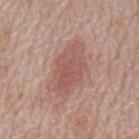Impression:
The lesion was tiled from a total-body skin photograph and was not biopsied.
Clinical summary:
The lesion's longest dimension is about 6.5 mm. On the mid back. Imaged with white-light lighting. A 15 mm crop from a total-body photograph taken for skin-cancer surveillance. The lesion-visualizer software estimated a shape eccentricity near 0.85 and a symmetry-axis asymmetry near 0.2. The software also gave a mean CIELAB color near L≈54 a*≈23 b*≈24, about 9 CIELAB-L* units darker than the surrounding skin, and a normalized lesion–skin contrast near 6.5. It also reported a peripheral color-asymmetry measure near 1. The analysis additionally found an automated nevus-likeness rating near 40 out of 100 and a lesion-detection confidence of about 100/100. A male subject, about 70 years old.